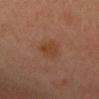biopsy status = total-body-photography surveillance lesion; no biopsy
subject = male, in their mid-30s
site = the head or neck
automated lesion analysis = an average lesion color of about L≈36 a*≈19 b*≈28 (CIELAB), a lesion–skin lightness drop of about 6, and a normalized lesion–skin contrast near 6.5; a border-irregularity index near 2/10, a color-variation rating of about 1.5/10, and radial color variation of about 0.5; an automated nevus-likeness rating near 15 out of 100 and a lesion-detection confidence of about 100/100
image source = 15 mm crop, total-body photography
tile lighting = cross-polarized illumination
diameter = ≈2.5 mm